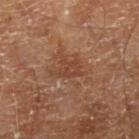biopsy_status: not biopsied; imaged during a skin examination
automated_metrics:
  border_irregularity_0_10: 4.5
  color_variation_0_10: 1.5
  peripheral_color_asymmetry: 0.5
patient:
  sex: male
  age_approx: 70
site: right lower leg
lighting: cross-polarized
image:
  source: total-body photography crop
  field_of_view_mm: 15